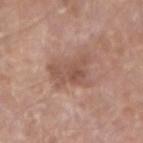Findings:
- follow-up — no biopsy performed (imaged during a skin exam)
- image — ~15 mm tile from a whole-body skin photo
- body site — the left forearm
- patient — male, about 65 years old